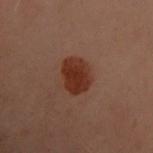Captured during whole-body skin photography for melanoma surveillance; the lesion was not biopsied.
A female patient in their 60s.
The lesion is located on the back.
The total-body-photography lesion software estimated an area of roughly 9.5 mm² and an outline eccentricity of about 0.55 (0 = round, 1 = elongated). The analysis additionally found about 9 CIELAB-L* units darker than the surrounding skin. It also reported a border-irregularity rating of about 1.5/10 and peripheral color asymmetry of about 0.5.
Cropped from a whole-body photographic skin survey; the tile spans about 15 mm.
The tile uses cross-polarized illumination.